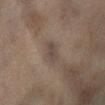biopsy_status: not biopsied; imaged during a skin examination
patient:
  sex: female
  age_approx: 60
site: left lower leg
image:
  source: total-body photography crop
  field_of_view_mm: 15
lesion_size:
  long_diameter_mm_approx: 3.0
lighting: cross-polarized